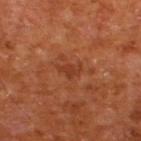- anatomic site — the upper back
- subject — male, approximately 65 years of age
- lighting — cross-polarized
- lesion size — about 2.5 mm
- image-analysis metrics — a shape eccentricity near 0.8 and a symmetry-axis asymmetry near 0.45; a lesion color around L≈36 a*≈28 b*≈34 in CIELAB, a lesion–skin lightness drop of about 7, and a lesion-to-skin contrast of about 5.5 (normalized; higher = more distinct); internal color variation of about 1 on a 0–10 scale and radial color variation of about 0.5
- image — ~15 mm tile from a whole-body skin photo